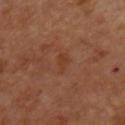Impression:
Captured during whole-body skin photography for melanoma surveillance; the lesion was not biopsied.
Image and clinical context:
Automated tile analysis of the lesion measured a footprint of about 2.5 mm² and two-axis asymmetry of about 0.4. It also reported a mean CIELAB color near L≈38 a*≈22 b*≈32 and a lesion–skin lightness drop of about 5. And it measured border irregularity of about 4.5 on a 0–10 scale, a within-lesion color-variation index near 0/10, and a peripheral color-asymmetry measure near 0. The analysis additionally found a nevus-likeness score of about 0/100 and a detector confidence of about 100 out of 100 that the crop contains a lesion. From the chest. The recorded lesion diameter is about 2.5 mm. Cropped from a total-body skin-imaging series; the visible field is about 15 mm. A male subject aged around 50.